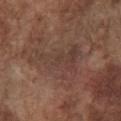  biopsy_status: not biopsied; imaged during a skin examination
  automated_metrics:
    area_mm2_approx: 16.0
    eccentricity: 0.8
    shape_asymmetry: 0.65
    nevus_likeness_0_100: 0
    lesion_detection_confidence_0_100: 60
  lighting: white-light
  patient:
    sex: male
    age_approx: 75
  lesion_size:
    long_diameter_mm_approx: 6.5
  image:
    source: total-body photography crop
    field_of_view_mm: 15
  site: chest